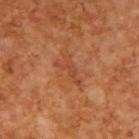Clinical impression:
Captured during whole-body skin photography for melanoma surveillance; the lesion was not biopsied.
Clinical summary:
Measured at roughly 4 mm in maximum diameter. A 15 mm crop from a total-body photograph taken for skin-cancer surveillance. This is a cross-polarized tile. The subject is a male aged 63–67.